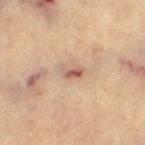{"biopsy_status": "not biopsied; imaged during a skin examination", "automated_metrics": {"area_mm2_approx": 3.5, "eccentricity": 0.8, "shape_asymmetry": 0.3, "cielab_L": 57, "cielab_a": 20, "cielab_b": 27, "vs_skin_darker_L": 11.0, "vs_skin_contrast_norm": 7.5, "border_irregularity_0_10": 3.0, "color_variation_0_10": 5.0, "nevus_likeness_0_100": 0, "lesion_detection_confidence_0_100": 100}, "lighting": "cross-polarized", "patient": {"sex": "female", "age_approx": 65}, "lesion_size": {"long_diameter_mm_approx": 2.5}, "image": {"source": "total-body photography crop", "field_of_view_mm": 15}, "site": "left thigh"}